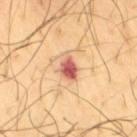Imaged during a routine full-body skin examination; the lesion was not biopsied and no histopathology is available. The lesion is on the right thigh. Automated tile analysis of the lesion measured a lesion area of about 5.5 mm², an outline eccentricity of about 0.7 (0 = round, 1 = elongated), and two-axis asymmetry of about 0.2. It also reported a classifier nevus-likeness of about 5/100. A male patient, aged around 65. This image is a 15 mm lesion crop taken from a total-body photograph. The lesion's longest dimension is about 3 mm.